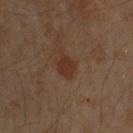– notes: total-body-photography surveillance lesion; no biopsy
– site: the right upper arm
– subject: male, about 60 years old
– lesion size: ~2.5 mm (longest diameter)
– illumination: cross-polarized illumination
– image: ~15 mm crop, total-body skin-cancer survey
– image-analysis metrics: a border-irregularity rating of about 3/10, a within-lesion color-variation index near 1/10, and a peripheral color-asymmetry measure near 0.5; a classifier nevus-likeness of about 65/100 and a detector confidence of about 100 out of 100 that the crop contains a lesion